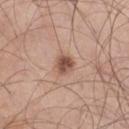This lesion was catalogued during total-body skin photography and was not selected for biopsy.
A male patient, aged 68 to 72.
About 2.5 mm across.
A 15 mm crop from a total-body photograph taken for skin-cancer surveillance.
From the right thigh.
This is a white-light tile.